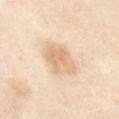The lesion was photographed on a routine skin check and not biopsied; there is no pathology result. On the front of the torso. This is a cross-polarized tile. Cropped from a whole-body photographic skin survey; the tile spans about 15 mm. A female subject aged around 55. Measured at roughly 4.5 mm in maximum diameter.